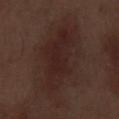biopsy_status: not biopsied; imaged during a skin examination
lighting: white-light
site: leg
image:
  source: total-body photography crop
  field_of_view_mm: 15
patient:
  sex: male
  age_approx: 70